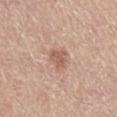This lesion was catalogued during total-body skin photography and was not selected for biopsy. A female subject aged around 65. The lesion is located on the left lower leg. This image is a 15 mm lesion crop taken from a total-body photograph.